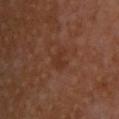Case summary:
- workup — total-body-photography surveillance lesion; no biopsy
- imaging modality — 15 mm crop, total-body photography
- lighting — cross-polarized
- body site — the back
- patient — male, aged 53 to 57
- diameter — ≈2.5 mm